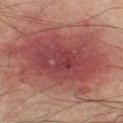This lesion was catalogued during total-body skin photography and was not selected for biopsy. Cropped from a whole-body photographic skin survey; the tile spans about 15 mm. A male patient, in their mid- to late 70s. The lesion is on the right thigh.